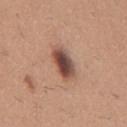Impression:
Captured during whole-body skin photography for melanoma surveillance; the lesion was not biopsied.
Background:
The lesion is located on the back. An algorithmic analysis of the crop reported a footprint of about 8 mm², a shape eccentricity near 0.8, and two-axis asymmetry of about 0.15. And it measured about 16 CIELAB-L* units darker than the surrounding skin. It also reported a classifier nevus-likeness of about 85/100 and a detector confidence of about 100 out of 100 that the crop contains a lesion. A male subject approximately 60 years of age. About 4 mm across. A 15 mm close-up extracted from a 3D total-body photography capture. Captured under white-light illumination.